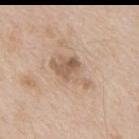follow-up = total-body-photography surveillance lesion; no biopsy
tile lighting = white-light illumination
image = 15 mm crop, total-body photography
diameter = about 5.5 mm
subject = male, roughly 65 years of age
site = the back